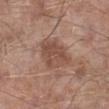Imaged during a routine full-body skin examination; the lesion was not biopsied and no histopathology is available. This is a white-light tile. The patient is a male approximately 55 years of age. A lesion tile, about 15 mm wide, cut from a 3D total-body photograph. Longest diameter approximately 4.5 mm. The lesion is located on the left lower leg.